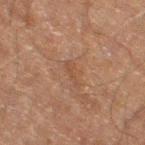Cropped from a whole-body photographic skin survey; the tile spans about 15 mm.
An algorithmic analysis of the crop reported an area of roughly 2.5 mm². It also reported a classifier nevus-likeness of about 0/100 and a detector confidence of about 100 out of 100 that the crop contains a lesion.
From the right thigh.
A male patient approximately 60 years of age.
The tile uses cross-polarized illumination.